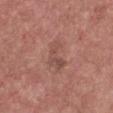Q: Is there a histopathology result?
A: no biopsy performed (imaged during a skin exam)
Q: Who is the patient?
A: female, aged around 45
Q: What lighting was used for the tile?
A: white-light
Q: What kind of image is this?
A: ~15 mm tile from a whole-body skin photo
Q: Where on the body is the lesion?
A: the chest